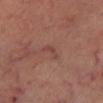Q: Was this lesion biopsied?
A: no biopsy performed (imaged during a skin exam)
Q: Patient demographics?
A: male, about 60 years old
Q: Where on the body is the lesion?
A: the left lower leg
Q: What is the imaging modality?
A: ~15 mm tile from a whole-body skin photo